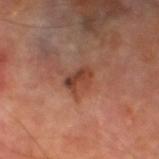Assessment: Part of a total-body skin-imaging series; this lesion was reviewed on a skin check and was not flagged for biopsy. Context: The lesion is located on the leg. Approximately 3 mm at its widest. A male subject, aged 68 to 72. A close-up tile cropped from a whole-body skin photograph, about 15 mm across. The tile uses cross-polarized illumination.